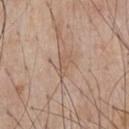This lesion was catalogued during total-body skin photography and was not selected for biopsy. The patient is a male in their 60s. The tile uses white-light illumination. On the chest. The lesion's longest dimension is about 2.5 mm. A roughly 15 mm field-of-view crop from a total-body skin photograph.